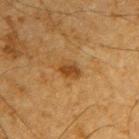Assessment: The lesion was tiled from a total-body skin photograph and was not biopsied. Image and clinical context: The lesion's longest dimension is about 2.5 mm. A lesion tile, about 15 mm wide, cut from a 3D total-body photograph. Imaged with cross-polarized lighting. The lesion is on the right upper arm. The subject is a male aged approximately 65. The lesion-visualizer software estimated an automated nevus-likeness rating near 70 out of 100 and lesion-presence confidence of about 100/100.